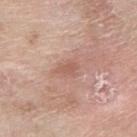Notes:
• workup: imaged on a skin check; not biopsied
• lesion size: about 2.5 mm
• site: the right forearm
• patient: female, aged 68 to 72
• illumination: white-light illumination
• acquisition: total-body-photography crop, ~15 mm field of view